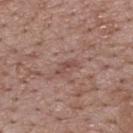• follow-up — no biopsy performed (imaged during a skin exam)
• diameter — ~3 mm (longest diameter)
• anatomic site — the back
• imaging modality — total-body-photography crop, ~15 mm field of view
• subject — male, aged around 70
• tile lighting — white-light
• TBP lesion metrics — a mean CIELAB color near L≈48 a*≈20 b*≈24 and about 8 CIELAB-L* units darker than the surrounding skin; a border-irregularity index near 6/10, internal color variation of about 0 on a 0–10 scale, and radial color variation of about 0